The lesion is located on the mid back · the lesion's longest dimension is about 10.5 mm · a 15 mm close-up tile from a total-body photography series done for melanoma screening · a male patient, aged around 55 · imaged with white-light lighting — 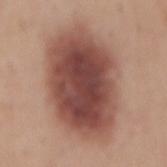Q: What did the biopsy show?
A: a dysplastic (Clark) nevus